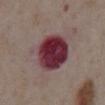{
  "biopsy_status": "not biopsied; imaged during a skin examination",
  "patient": {
    "sex": "male",
    "age_approx": 75
  },
  "site": "abdomen",
  "lighting": "white-light",
  "lesion_size": {
    "long_diameter_mm_approx": 5.5
  },
  "image": {
    "source": "total-body photography crop",
    "field_of_view_mm": 15
  }
}